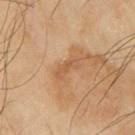Recorded during total-body skin imaging; not selected for excision or biopsy.
This image is a 15 mm lesion crop taken from a total-body photograph.
Automated tile analysis of the lesion measured a mean CIELAB color near L≈57 a*≈21 b*≈37, about 7 CIELAB-L* units darker than the surrounding skin, and a normalized border contrast of about 5. The software also gave border irregularity of about 4 on a 0–10 scale, a color-variation rating of about 1.5/10, and a peripheral color-asymmetry measure near 0.5. The software also gave an automated nevus-likeness rating near 0 out of 100 and lesion-presence confidence of about 100/100.
On the right upper arm.
The tile uses cross-polarized illumination.
Measured at roughly 3.5 mm in maximum diameter.
A male subject, aged 63–67.